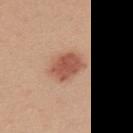Part of a total-body skin-imaging series; this lesion was reviewed on a skin check and was not flagged for biopsy. Approximately 4 mm at its widest. Cropped from a whole-body photographic skin survey; the tile spans about 15 mm. The subject is a female about 25 years old. Imaged with white-light lighting. From the upper back.